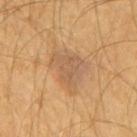Clinical impression:
Captured during whole-body skin photography for melanoma surveillance; the lesion was not biopsied.
Acquisition and patient details:
On the mid back. A male subject, aged approximately 60. A lesion tile, about 15 mm wide, cut from a 3D total-body photograph. Longest diameter approximately 5.5 mm.